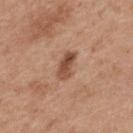Part of a total-body skin-imaging series; this lesion was reviewed on a skin check and was not flagged for biopsy.
The lesion is located on the upper back.
A close-up tile cropped from a whole-body skin photograph, about 15 mm across.
Measured at roughly 3.5 mm in maximum diameter.
Captured under white-light illumination.
A female patient, aged 38 to 42.